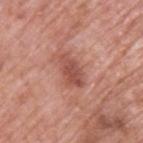The lesion was photographed on a routine skin check and not biopsied; there is no pathology result. Cropped from a total-body skin-imaging series; the visible field is about 15 mm. The lesion's longest dimension is about 4 mm. The lesion is on the upper back. A male subject, in their 70s. Imaged with white-light lighting. Automated tile analysis of the lesion measured an area of roughly 6.5 mm² and two-axis asymmetry of about 0.25.